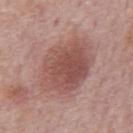Q: Is there a histopathology result?
A: total-body-photography surveillance lesion; no biopsy
Q: Lesion size?
A: ~8 mm (longest diameter)
Q: Who is the patient?
A: male, aged approximately 75
Q: What is the imaging modality?
A: ~15 mm crop, total-body skin-cancer survey
Q: Lesion location?
A: the mid back